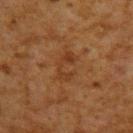Q: Is there a histopathology result?
A: total-body-photography surveillance lesion; no biopsy
Q: What are the patient's age and sex?
A: male, roughly 60 years of age
Q: How was the tile lit?
A: cross-polarized
Q: How was this image acquired?
A: ~15 mm tile from a whole-body skin photo
Q: What is the anatomic site?
A: the upper back
Q: Lesion size?
A: about 3.5 mm